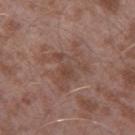  image:
    source: total-body photography crop
    field_of_view_mm: 15
  patient:
    sex: male
    age_approx: 45
  lighting: white-light
  site: left thigh
  automated_metrics:
    vs_skin_darker_L: 6.0
    vs_skin_contrast_norm: 5.5
  lesion_size:
    long_diameter_mm_approx: 5.0